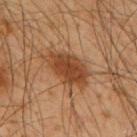Captured during whole-body skin photography for melanoma surveillance; the lesion was not biopsied.
The lesion is located on the upper back.
A roughly 15 mm field-of-view crop from a total-body skin photograph.
A male patient in their mid-60s.